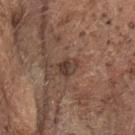follow-up: no biopsy performed (imaged during a skin exam) | acquisition: 15 mm crop, total-body photography | body site: the head or neck | subject: male, in their mid-70s.